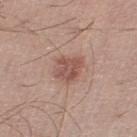Assessment:
Part of a total-body skin-imaging series; this lesion was reviewed on a skin check and was not flagged for biopsy.
Clinical summary:
A male patient, aged 18–22. The lesion is on the left lower leg. The tile uses white-light illumination. Cropped from a total-body skin-imaging series; the visible field is about 15 mm. Approximately 3 mm at its widest.